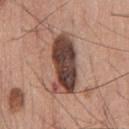• workup · catalogued during a skin exam; not biopsied
• site · the chest
• imaging modality · total-body-photography crop, ~15 mm field of view
• patient · male, approximately 60 years of age